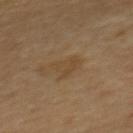notes: catalogued during a skin exam; not biopsied | patient: male, aged 58–62 | image-analysis metrics: a border-irregularity rating of about 4.5/10 and peripheral color asymmetry of about 0.5; a nevus-likeness score of about 0/100 and a lesion-detection confidence of about 100/100 | body site: the back | size: about 2.5 mm | image: ~15 mm tile from a whole-body skin photo.